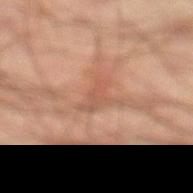{"biopsy_status": "not biopsied; imaged during a skin examination", "patient": {"sex": "male", "age_approx": 45}, "site": "left lower leg", "image": {"source": "total-body photography crop", "field_of_view_mm": 15}, "automated_metrics": {"border_irregularity_0_10": 5.5, "color_variation_0_10": 2.0}}